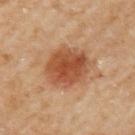Notes:
* biopsy status — no biopsy performed (imaged during a skin exam)
* diameter — about 5 mm
* body site — the left upper arm
* lighting — cross-polarized
* image-analysis metrics — a footprint of about 18 mm², an outline eccentricity of about 0.4 (0 = round, 1 = elongated), and a symmetry-axis asymmetry near 0.1; an average lesion color of about L≈50 a*≈25 b*≈36 (CIELAB) and a lesion–skin lightness drop of about 12; a border-irregularity rating of about 1.5/10, a within-lesion color-variation index near 4.5/10, and peripheral color asymmetry of about 1.5; a nevus-likeness score of about 100/100 and a lesion-detection confidence of about 100/100
* image source — ~15 mm crop, total-body skin-cancer survey
* subject — female, aged around 60